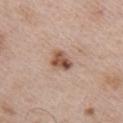Q: Was a biopsy performed?
A: total-body-photography surveillance lesion; no biopsy